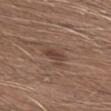Imaged during a routine full-body skin examination; the lesion was not biopsied and no histopathology is available.